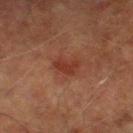Findings:
• biopsy status · no biopsy performed (imaged during a skin exam)
• subject · male, aged 73 to 77
• lesion size · ≈3 mm
• tile lighting · cross-polarized illumination
• TBP lesion metrics · an average lesion color of about L≈29 a*≈23 b*≈26 (CIELAB), about 7 CIELAB-L* units darker than the surrounding skin, and a normalized lesion–skin contrast near 7; a border-irregularity rating of about 3.5/10 and internal color variation of about 1 on a 0–10 scale; a classifier nevus-likeness of about 10/100 and lesion-presence confidence of about 100/100
• acquisition · 15 mm crop, total-body photography
• anatomic site · the leg